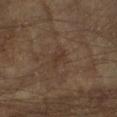Assessment: The lesion was tiled from a total-body skin photograph and was not biopsied. Background: The lesion is on the right forearm. A male subject roughly 65 years of age. Imaged with cross-polarized lighting. A lesion tile, about 15 mm wide, cut from a 3D total-body photograph.